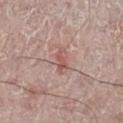– location: the leg
– lesion diameter: ~3 mm (longest diameter)
– patient: male, aged around 70
– TBP lesion metrics: a shape eccentricity near 0.9 and a shape-asymmetry score of about 0.4 (0 = symmetric)
– acquisition: ~15 mm crop, total-body skin-cancer survey
– tile lighting: white-light